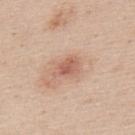- workup: catalogued during a skin exam; not biopsied
- tile lighting: white-light
- image-analysis metrics: a lesion area of about 5 mm², an outline eccentricity of about 0.8 (0 = round, 1 = elongated), and two-axis asymmetry of about 0.3; an average lesion color of about L≈60 a*≈23 b*≈29 (CIELAB), a lesion–skin lightness drop of about 10, and a lesion-to-skin contrast of about 6.5 (normalized; higher = more distinct); a color-variation rating of about 3/10 and a peripheral color-asymmetry measure near 1; a lesion-detection confidence of about 100/100
- patient: male, roughly 45 years of age
- size: about 3.5 mm
- anatomic site: the upper back
- image: 15 mm crop, total-body photography